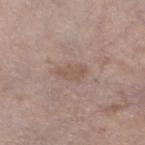Q: Was a biopsy performed?
A: total-body-photography surveillance lesion; no biopsy
Q: What kind of image is this?
A: ~15 mm crop, total-body skin-cancer survey
Q: What is the anatomic site?
A: the left lower leg
Q: What lighting was used for the tile?
A: white-light
Q: Who is the patient?
A: male, approximately 60 years of age